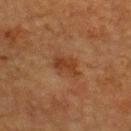Q: Was this lesion biopsied?
A: imaged on a skin check; not biopsied
Q: What did automated image analysis measure?
A: an average lesion color of about L≈32 a*≈20 b*≈30 (CIELAB), roughly 7 lightness units darker than nearby skin, and a normalized lesion–skin contrast near 7; border irregularity of about 4 on a 0–10 scale, internal color variation of about 2 on a 0–10 scale, and radial color variation of about 0.5; a classifier nevus-likeness of about 10/100
Q: How was the tile lit?
A: cross-polarized
Q: What is the lesion's diameter?
A: ≈3.5 mm
Q: Where on the body is the lesion?
A: the upper back
Q: What are the patient's age and sex?
A: female, roughly 55 years of age
Q: What is the imaging modality?
A: total-body-photography crop, ~15 mm field of view